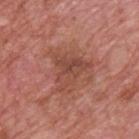Background:
A male patient, approximately 75 years of age. The lesion is on the upper back. A region of skin cropped from a whole-body photographic capture, roughly 15 mm wide. Imaged with white-light lighting.